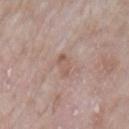Assessment:
No biopsy was performed on this lesion — it was imaged during a full skin examination and was not determined to be concerning.
Clinical summary:
Captured under white-light illumination. The subject is a male roughly 65 years of age. On the left upper arm. Measured at roughly 3 mm in maximum diameter. Cropped from a total-body skin-imaging series; the visible field is about 15 mm.